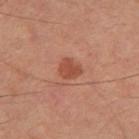TBP lesion metrics: a shape eccentricity near 0.45 and two-axis asymmetry of about 0.2; a lesion–skin lightness drop of about 9; a nevus-likeness score of about 95/100 and lesion-presence confidence of about 100/100
site: the left thigh
lesion size: ~2.5 mm (longest diameter)
subject: male, in their mid-60s
image: ~15 mm crop, total-body skin-cancer survey
illumination: cross-polarized